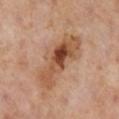Clinical impression: This lesion was catalogued during total-body skin photography and was not selected for biopsy. Context: The lesion-visualizer software estimated an average lesion color of about L≈52 a*≈22 b*≈33 (CIELAB), roughly 12 lightness units darker than nearby skin, and a lesion-to-skin contrast of about 9 (normalized; higher = more distinct). And it measured border irregularity of about 4 on a 0–10 scale, a within-lesion color-variation index near 9.5/10, and radial color variation of about 3. From the right lower leg. A 15 mm close-up tile from a total-body photography series done for melanoma screening. A female patient in their mid- to late 50s.